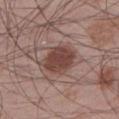Part of a total-body skin-imaging series; this lesion was reviewed on a skin check and was not flagged for biopsy. A male patient, aged approximately 55. From the left lower leg. A roughly 15 mm field-of-view crop from a total-body skin photograph.